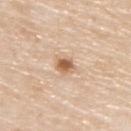Impression: Captured during whole-body skin photography for melanoma surveillance; the lesion was not biopsied. Clinical summary: The tile uses white-light illumination. A 15 mm close-up tile from a total-body photography series done for melanoma screening. A male subject, aged 78 to 82. The lesion's longest dimension is about 3 mm. The lesion is located on the upper back.